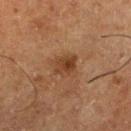Q: Was this lesion biopsied?
A: imaged on a skin check; not biopsied
Q: Lesion size?
A: ≈2.5 mm
Q: Who is the patient?
A: male, approximately 65 years of age
Q: Lesion location?
A: the left lower leg
Q: What kind of image is this?
A: total-body-photography crop, ~15 mm field of view